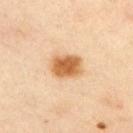Impression: This lesion was catalogued during total-body skin photography and was not selected for biopsy. Background: A male patient, roughly 45 years of age. On the right upper arm. Longest diameter approximately 4 mm. A roughly 15 mm field-of-view crop from a total-body skin photograph. An algorithmic analysis of the crop reported a border-irregularity index near 1.5/10, a color-variation rating of about 4/10, and peripheral color asymmetry of about 1. And it measured an automated nevus-likeness rating near 100 out of 100. This is a cross-polarized tile.